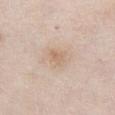workup = imaged on a skin check; not biopsied | tile lighting = white-light illumination | site = the front of the torso | diameter = about 3 mm | imaging modality = 15 mm crop, total-body photography | patient = female, aged 58 to 62.